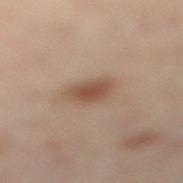Captured under cross-polarized illumination.
A close-up tile cropped from a whole-body skin photograph, about 15 mm across.
The lesion-visualizer software estimated a footprint of about 6 mm² and an outline eccentricity of about 0.6 (0 = round, 1 = elongated). It also reported a within-lesion color-variation index near 2.5/10.
About 3 mm across.
The lesion is located on the left leg.
A female subject aged 53 to 57.